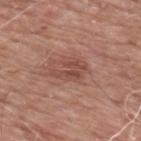This lesion was catalogued during total-body skin photography and was not selected for biopsy. About 4 mm across. Located on the upper back. The tile uses white-light illumination. A 15 mm close-up extracted from a 3D total-body photography capture. The patient is a male about 60 years old.